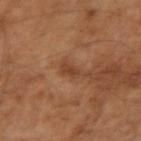Context:
This is a cross-polarized tile. A lesion tile, about 15 mm wide, cut from a 3D total-body photograph. On the left arm. Longest diameter approximately 2.5 mm. A male patient, in their mid- to late 60s.